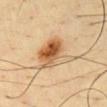Clinical summary:
A male subject, roughly 45 years of age. The tile uses cross-polarized illumination. The lesion-visualizer software estimated a lesion area of about 14 mm², an eccentricity of roughly 0.5, and a shape-asymmetry score of about 0.3 (0 = symmetric). And it measured a classifier nevus-likeness of about 95/100 and a detector confidence of about 100 out of 100 that the crop contains a lesion. The recorded lesion diameter is about 5 mm. The lesion is located on the chest. Cropped from a total-body skin-imaging series; the visible field is about 15 mm.